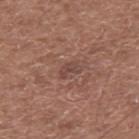<record>
  <biopsy_status>not biopsied; imaged during a skin examination</biopsy_status>
  <patient>
    <sex>male</sex>
    <age_approx>60</age_approx>
  </patient>
  <site>back</site>
  <image>
    <source>total-body photography crop</source>
    <field_of_view_mm>15</field_of_view_mm>
  </image>
  <lesion_size>
    <long_diameter_mm_approx>2.5</long_diameter_mm_approx>
  </lesion_size>
</record>